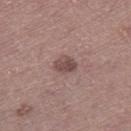<tbp_lesion>
<biopsy_status>not biopsied; imaged during a skin examination</biopsy_status>
<image>
  <source>total-body photography crop</source>
  <field_of_view_mm>15</field_of_view_mm>
</image>
<patient>
  <sex>male</sex>
  <age_approx>45</age_approx>
</patient>
<lighting>white-light</lighting>
<lesion_size>
  <long_diameter_mm_approx>2.5</long_diameter_mm_approx>
</lesion_size>
<site>right thigh</site>
</tbp_lesion>